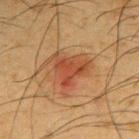Notes:
- patient: male, aged approximately 35
- size: ~5 mm (longest diameter)
- site: the right upper arm
- image: ~15 mm crop, total-body skin-cancer survey
- tile lighting: cross-polarized illumination